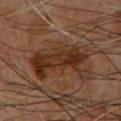{
  "biopsy_status": "not biopsied; imaged during a skin examination",
  "image": {
    "source": "total-body photography crop",
    "field_of_view_mm": 15
  },
  "lesion_size": {
    "long_diameter_mm_approx": 7.5
  },
  "lighting": "cross-polarized",
  "patient": {
    "sex": "male",
    "age_approx": 60
  },
  "site": "back"
}